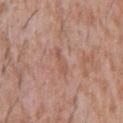image: 15 mm crop, total-body photography | tile lighting: white-light illumination | anatomic site: the front of the torso | TBP lesion metrics: an area of roughly 2.5 mm² and a symmetry-axis asymmetry near 0.4; a border-irregularity rating of about 4.5/10, internal color variation of about 0 on a 0–10 scale, and a peripheral color-asymmetry measure near 0 | diameter: ≈3 mm | patient: male, in their mid- to late 40s.